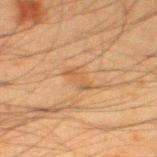The lesion was photographed on a routine skin check and not biopsied; there is no pathology result. Located on the left thigh. Automated image analysis of the tile measured a lesion–skin lightness drop of about 6 and a lesion-to-skin contrast of about 5.5 (normalized; higher = more distinct). Cropped from a whole-body photographic skin survey; the tile spans about 15 mm. A male subject, approximately 35 years of age. This is a cross-polarized tile. Approximately 3 mm at its widest.